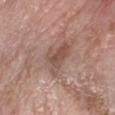{
  "lighting": "white-light",
  "site": "right forearm",
  "patient": {
    "sex": "female",
    "age_approx": 75
  },
  "lesion_size": {
    "long_diameter_mm_approx": 4.0
  },
  "image": {
    "source": "total-body photography crop",
    "field_of_view_mm": 15
  }
}